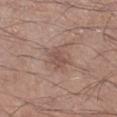| feature | finding |
|---|---|
| follow-up | total-body-photography surveillance lesion; no biopsy |
| anatomic site | the leg |
| patient | male, aged approximately 55 |
| lighting | white-light |
| automated lesion analysis | an eccentricity of roughly 0.7; a lesion color around L≈51 a*≈18 b*≈24 in CIELAB, roughly 7 lightness units darker than nearby skin, and a normalized lesion–skin contrast near 5.5; an automated nevus-likeness rating near 0 out of 100 and lesion-presence confidence of about 100/100 |
| image source | ~15 mm crop, total-body skin-cancer survey |